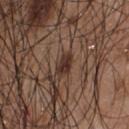follow-up — catalogued during a skin exam; not biopsied
lesion size — ~3 mm (longest diameter)
patient — male, in their mid-50s
image source — total-body-photography crop, ~15 mm field of view
site — the chest